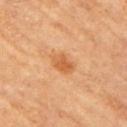Impression:
This lesion was catalogued during total-body skin photography and was not selected for biopsy.
Acquisition and patient details:
Located on the left arm. The recorded lesion diameter is about 3 mm. The subject is a female aged 63 to 67. This image is a 15 mm lesion crop taken from a total-body photograph.